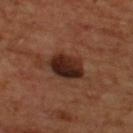  biopsy_status: not biopsied; imaged during a skin examination
  patient:
    sex: male
    age_approx: 70
  image:
    source: total-body photography crop
    field_of_view_mm: 15
  automated_metrics:
    area_mm2_approx: 10.0
    eccentricity: 0.65
    shape_asymmetry: 0.15
    cielab_L: 24
    cielab_a: 20
    cielab_b: 24
    vs_skin_contrast_norm: 13.0
    border_irregularity_0_10: 1.5
    color_variation_0_10: 6.0
    peripheral_color_asymmetry: 2.0
  lighting: cross-polarized
  site: upper back
  lesion_size:
    long_diameter_mm_approx: 4.0